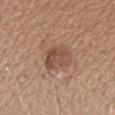{
  "image": {
    "source": "total-body photography crop",
    "field_of_view_mm": 15
  },
  "lighting": "white-light",
  "site": "arm",
  "lesion_size": {
    "long_diameter_mm_approx": 4.5
  },
  "automated_metrics": {
    "area_mm2_approx": 10.0,
    "eccentricity": 0.8,
    "shape_asymmetry": 0.2,
    "vs_skin_darker_L": 9.0,
    "vs_skin_contrast_norm": 6.5,
    "nevus_likeness_0_100": 55,
    "lesion_detection_confidence_0_100": 100
  },
  "patient": {
    "sex": "female",
    "age_approx": 35
  }
}